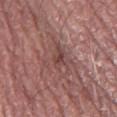This lesion was catalogued during total-body skin photography and was not selected for biopsy. The recorded lesion diameter is about 5 mm. Automated image analysis of the tile measured a within-lesion color-variation index near 6/10 and a peripheral color-asymmetry measure near 2. On the leg. Imaged with white-light lighting. A region of skin cropped from a whole-body photographic capture, roughly 15 mm wide. A male subject, roughly 60 years of age.